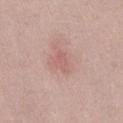The lesion was photographed on a routine skin check and not biopsied; there is no pathology result.
A roughly 15 mm field-of-view crop from a total-body skin photograph.
Automated image analysis of the tile measured an automated nevus-likeness rating near 0 out of 100 and a detector confidence of about 100 out of 100 that the crop contains a lesion.
A female patient, aged 18–22.
From the right thigh.
This is a white-light tile.
Longest diameter approximately 2.5 mm.